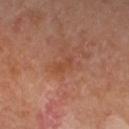{
  "biopsy_status": "not biopsied; imaged during a skin examination",
  "image": {
    "source": "total-body photography crop",
    "field_of_view_mm": 15
  },
  "lesion_size": {
    "long_diameter_mm_approx": 2.5
  },
  "site": "right forearm",
  "lighting": "cross-polarized",
  "patient": {
    "sex": "female",
    "age_approx": 55
  },
  "automated_metrics": {
    "area_mm2_approx": 2.5,
    "eccentricity": 0.9,
    "cielab_L": 47,
    "cielab_a": 25,
    "cielab_b": 33,
    "vs_skin_darker_L": 5.0,
    "vs_skin_contrast_norm": 5.0,
    "border_irregularity_0_10": 3.5,
    "peripheral_color_asymmetry": 0.0,
    "nevus_likeness_0_100": 0,
    "lesion_detection_confidence_0_100": 100
  }
}